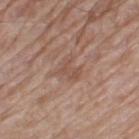Part of a total-body skin-imaging series; this lesion was reviewed on a skin check and was not flagged for biopsy. The subject is a male about 80 years old. This image is a 15 mm lesion crop taken from a total-body photograph. The lesion is located on the left thigh. Measured at roughly 3 mm in maximum diameter.